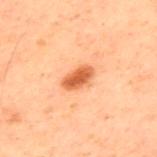| key | value |
|---|---|
| follow-up | catalogued during a skin exam; not biopsied |
| body site | the upper back |
| image | 15 mm crop, total-body photography |
| subject | male, roughly 60 years of age |